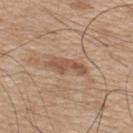Assessment:
The lesion was photographed on a routine skin check and not biopsied; there is no pathology result.
Background:
The lesion is located on the upper back. Automated image analysis of the tile measured a lesion area of about 6 mm², an eccentricity of roughly 0.95, and two-axis asymmetry of about 0.45. It also reported a border-irregularity rating of about 5.5/10 and radial color variation of about 0.5. The patient is a male in their mid- to late 70s. The tile uses white-light illumination. Cropped from a total-body skin-imaging series; the visible field is about 15 mm.